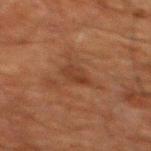The lesion was tiled from a total-body skin photograph and was not biopsied.
The total-body-photography lesion software estimated a lesion-to-skin contrast of about 6 (normalized; higher = more distinct). And it measured an automated nevus-likeness rating near 0 out of 100 and lesion-presence confidence of about 100/100.
The tile uses cross-polarized illumination.
The lesion is located on the upper back.
Cropped from a whole-body photographic skin survey; the tile spans about 15 mm.
A male subject aged 58 to 62.
About 2.5 mm across.